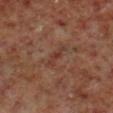No biopsy was performed on this lesion — it was imaged during a full skin examination and was not determined to be concerning.
The lesion is located on the right lower leg.
A region of skin cropped from a whole-body photographic capture, roughly 15 mm wide.
A male patient, in their 60s.